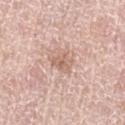This lesion was catalogued during total-body skin photography and was not selected for biopsy.
The lesion's longest dimension is about 2.5 mm.
Captured under white-light illumination.
The lesion is located on the left thigh.
Cropped from a total-body skin-imaging series; the visible field is about 15 mm.
The total-body-photography lesion software estimated an average lesion color of about L≈63 a*≈19 b*≈27 (CIELAB), a lesion–skin lightness drop of about 9, and a lesion-to-skin contrast of about 5.5 (normalized; higher = more distinct). And it measured border irregularity of about 2.5 on a 0–10 scale, internal color variation of about 2.5 on a 0–10 scale, and a peripheral color-asymmetry measure near 1. The software also gave a classifier nevus-likeness of about 0/100.
The patient is a female aged 58 to 62.